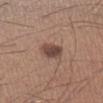Clinical summary:
The lesion is on the right lower leg. The subject is a male in their mid- to late 30s. An algorithmic analysis of the crop reported a border-irregularity rating of about 2/10 and peripheral color asymmetry of about 1. And it measured lesion-presence confidence of about 100/100. Longest diameter approximately 2.5 mm. Captured under white-light illumination. A 15 mm close-up tile from a total-body photography series done for melanoma screening.